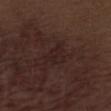Q: Was a biopsy performed?
A: no biopsy performed (imaged during a skin exam)
Q: Where on the body is the lesion?
A: the abdomen
Q: Patient demographics?
A: male, about 70 years old
Q: What lighting was used for the tile?
A: white-light illumination
Q: What kind of image is this?
A: 15 mm crop, total-body photography
Q: Automated lesion metrics?
A: an area of roughly 5 mm² and a symmetry-axis asymmetry near 0.4; a lesion color around L≈21 a*≈16 b*≈16 in CIELAB and about 4 CIELAB-L* units darker than the surrounding skin; a within-lesion color-variation index near 2/10 and a peripheral color-asymmetry measure near 0.5; a nevus-likeness score of about 0/100 and a lesion-detection confidence of about 95/100